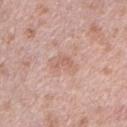The lesion's longest dimension is about 3 mm. The lesion is on the right upper arm. The subject is a male aged 38–42. A roughly 15 mm field-of-view crop from a total-body skin photograph.